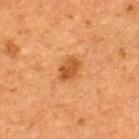Imaged during a routine full-body skin examination; the lesion was not biopsied and no histopathology is available. The tile uses cross-polarized illumination. From the upper back. A region of skin cropped from a whole-body photographic capture, roughly 15 mm wide. Longest diameter approximately 3 mm. The patient is a male roughly 50 years of age. Automated image analysis of the tile measured a lesion area of about 5 mm² and two-axis asymmetry of about 0.25. The analysis additionally found a nevus-likeness score of about 85/100 and a lesion-detection confidence of about 100/100.